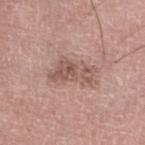Q: Was this lesion biopsied?
A: total-body-photography surveillance lesion; no biopsy